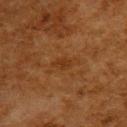site = the upper back | lighting = cross-polarized illumination | TBP lesion metrics = a border-irregularity index near 2/10, a within-lesion color-variation index near 1.5/10, and a peripheral color-asymmetry measure near 0.5; a nevus-likeness score of about 0/100 and lesion-presence confidence of about 100/100 | acquisition = ~15 mm crop, total-body skin-cancer survey | patient = female, in their 50s.